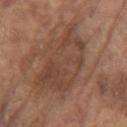Imaged during a routine full-body skin examination; the lesion was not biopsied and no histopathology is available. A male subject aged 78 to 82. The lesion is located on the left upper arm. Cropped from a whole-body photographic skin survey; the tile spans about 15 mm.